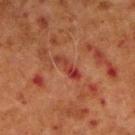workup — no biopsy performed (imaged during a skin exam)
image source — total-body-photography crop, ~15 mm field of view
patient — male, aged around 70
body site — the right upper arm